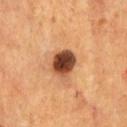Recorded during total-body skin imaging; not selected for excision or biopsy. The total-body-photography lesion software estimated lesion-presence confidence of about 100/100. A male subject, approximately 55 years of age. The recorded lesion diameter is about 4 mm. The tile uses cross-polarized illumination. A lesion tile, about 15 mm wide, cut from a 3D total-body photograph. On the chest.